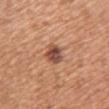Part of a total-body skin-imaging series; this lesion was reviewed on a skin check and was not flagged for biopsy.
Approximately 3 mm at its widest.
A close-up tile cropped from a whole-body skin photograph, about 15 mm across.
Located on the chest.
The patient is a female about 55 years old.